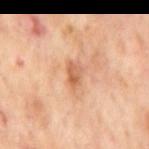Notes:
– follow-up · no biopsy performed (imaged during a skin exam)
– location · the mid back
– tile lighting · cross-polarized illumination
– acquisition · ~15 mm crop, total-body skin-cancer survey
– subject · male, aged 63–67